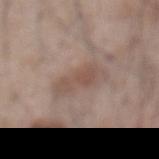Recorded during total-body skin imaging; not selected for excision or biopsy.
A male subject about 55 years old.
The lesion's longest dimension is about 4 mm.
The total-body-photography lesion software estimated an average lesion color of about L≈50 a*≈17 b*≈24 (CIELAB) and a lesion-to-skin contrast of about 6 (normalized; higher = more distinct). It also reported internal color variation of about 1.5 on a 0–10 scale and a peripheral color-asymmetry measure near 0.5. It also reported a nevus-likeness score of about 10/100 and a detector confidence of about 100 out of 100 that the crop contains a lesion.
A 15 mm close-up extracted from a 3D total-body photography capture.
The lesion is on the front of the torso.